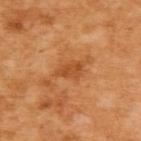workup = total-body-photography surveillance lesion; no biopsy | patient = female, aged around 55 | anatomic site = the upper back | automated metrics = border irregularity of about 3 on a 0–10 scale and radial color variation of about 0.5; lesion-presence confidence of about 100/100 | tile lighting = cross-polarized | image = ~15 mm crop, total-body skin-cancer survey.